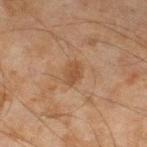lighting: cross-polarized
imaging modality: 15 mm crop, total-body photography
body site: the leg
subject: male, in their mid-40s